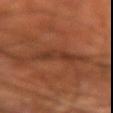{"image": {"source": "total-body photography crop", "field_of_view_mm": 15}, "lighting": "cross-polarized", "site": "left forearm", "patient": {"sex": "male", "age_approx": 55}, "lesion_size": {"long_diameter_mm_approx": 4.0}}